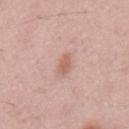Clinical impression:
The lesion was photographed on a routine skin check and not biopsied; there is no pathology result.
Image and clinical context:
A 15 mm crop from a total-body photograph taken for skin-cancer surveillance. The patient is a male aged approximately 55.